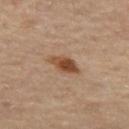notes: catalogued during a skin exam; not biopsied
site: the left thigh
image: ~15 mm crop, total-body skin-cancer survey
tile lighting: cross-polarized illumination
TBP lesion metrics: a lesion color around L≈47 a*≈21 b*≈32 in CIELAB and roughly 12 lightness units darker than nearby skin; a border-irregularity index near 2/10, a within-lesion color-variation index near 5/10, and a peripheral color-asymmetry measure near 2; an automated nevus-likeness rating near 95 out of 100 and a detector confidence of about 100 out of 100 that the crop contains a lesion
subject: female, in their mid-50s
lesion size: ≈3.5 mm